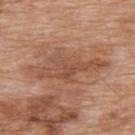biopsy status: no biopsy performed (imaged during a skin exam) | TBP lesion metrics: an outline eccentricity of about 0.95 (0 = round, 1 = elongated); a classifier nevus-likeness of about 0/100 and a lesion-detection confidence of about 60/100 | image source: 15 mm crop, total-body photography | diameter: ~9 mm (longest diameter) | location: the upper back | subject: male, roughly 80 years of age | tile lighting: white-light.